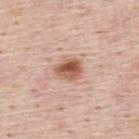follow-up: imaged on a skin check; not biopsied